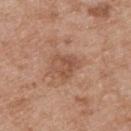| field | value |
|---|---|
| workup | no biopsy performed (imaged during a skin exam) |
| site | the upper back |
| lesion size | about 3 mm |
| patient | male, aged 53 to 57 |
| illumination | white-light illumination |
| acquisition | 15 mm crop, total-body photography |
| automated lesion analysis | a mean CIELAB color near L≈52 a*≈21 b*≈30, about 8 CIELAB-L* units darker than the surrounding skin, and a lesion-to-skin contrast of about 6 (normalized; higher = more distinct); a border-irregularity index near 3.5/10, internal color variation of about 4 on a 0–10 scale, and a peripheral color-asymmetry measure near 1.5 |